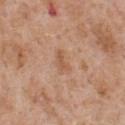biopsy status: imaged on a skin check; not biopsied
subject: male, in their mid-60s
tile lighting: white-light illumination
automated lesion analysis: an area of roughly 3 mm²; a mean CIELAB color near L≈56 a*≈21 b*≈33 and a lesion-to-skin contrast of about 5.5 (normalized; higher = more distinct); border irregularity of about 4 on a 0–10 scale and a color-variation rating of about 0.5/10
imaging modality: 15 mm crop, total-body photography
body site: the front of the torso
diameter: ~3 mm (longest diameter)